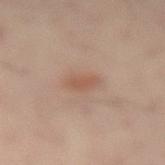Case summary:
– follow-up · total-body-photography surveillance lesion; no biopsy
– tile lighting · cross-polarized
– body site · the lower back
– acquisition · 15 mm crop, total-body photography
– subject · male, aged approximately 40
– diameter · about 2.5 mm